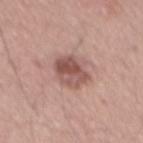tile lighting = white-light
diameter = about 4 mm
image source = ~15 mm crop, total-body skin-cancer survey
site = the mid back
patient = male, in their mid-50s